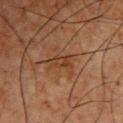Captured during whole-body skin photography for melanoma surveillance; the lesion was not biopsied.
From the chest.
A male subject, about 65 years old.
A lesion tile, about 15 mm wide, cut from a 3D total-body photograph.